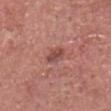workup=catalogued during a skin exam; not biopsied
automated lesion analysis=an average lesion color of about L≈48 a*≈25 b*≈24 (CIELAB) and a normalized border contrast of about 7; a nevus-likeness score of about 5/100 and a lesion-detection confidence of about 100/100
subject=male, aged 78–82
illumination=white-light
imaging modality=total-body-photography crop, ~15 mm field of view
location=the head or neck
size=about 2.5 mm